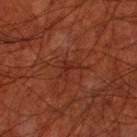follow-up = total-body-photography surveillance lesion; no biopsy
body site = the left thigh
lesion size = about 2.5 mm
illumination = cross-polarized
subject = male, aged 68–72
image source = total-body-photography crop, ~15 mm field of view